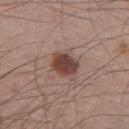Impression: Part of a total-body skin-imaging series; this lesion was reviewed on a skin check and was not flagged for biopsy. Context: Imaged with white-light lighting. Measured at roughly 3.5 mm in maximum diameter. Cropped from a whole-body photographic skin survey; the tile spans about 15 mm. An algorithmic analysis of the crop reported an average lesion color of about L≈42 a*≈20 b*≈23 (CIELAB), about 14 CIELAB-L* units darker than the surrounding skin, and a normalized lesion–skin contrast near 11. The software also gave a classifier nevus-likeness of about 95/100. From the leg. A male patient, aged approximately 45.